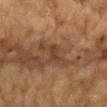Impression: Imaged during a routine full-body skin examination; the lesion was not biopsied and no histopathology is available. Image and clinical context: This is a cross-polarized tile. A 15 mm close-up extracted from a 3D total-body photography capture. From the chest. The subject is a male aged 83 or older.